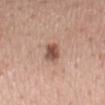Acquisition and patient details: The lesion-visualizer software estimated an area of roughly 5 mm², an outline eccentricity of about 0.65 (0 = round, 1 = elongated), and two-axis asymmetry of about 0.3. The analysis additionally found an average lesion color of about L≈52 a*≈21 b*≈26 (CIELAB), a lesion–skin lightness drop of about 13, and a lesion-to-skin contrast of about 9 (normalized; higher = more distinct). The analysis additionally found a border-irregularity rating of about 2.5/10, internal color variation of about 5.5 on a 0–10 scale, and peripheral color asymmetry of about 2. Cropped from a whole-body photographic skin survey; the tile spans about 15 mm. The patient is a female aged approximately 60. The lesion's longest dimension is about 3 mm. From the back.